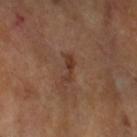Notes:
• follow-up · total-body-photography surveillance lesion; no biopsy
• image · 15 mm crop, total-body photography
• subject · female, aged 73 to 77
• illumination · cross-polarized illumination
• lesion diameter · ≈3 mm
• automated metrics · a color-variation rating of about 0.5/10; a nevus-likeness score of about 5/100 and lesion-presence confidence of about 100/100
• body site · the arm